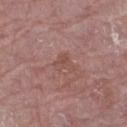Clinical impression: This lesion was catalogued during total-body skin photography and was not selected for biopsy. Clinical summary: Imaged with white-light lighting. The lesion is located on the left thigh. The total-body-photography lesion software estimated a lesion color around L≈49 a*≈22 b*≈24 in CIELAB, roughly 6 lightness units darker than nearby skin, and a lesion-to-skin contrast of about 5 (normalized; higher = more distinct). Longest diameter approximately 2.5 mm. Cropped from a whole-body photographic skin survey; the tile spans about 15 mm. A female patient in their mid-60s.